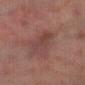Part of a total-body skin-imaging series; this lesion was reviewed on a skin check and was not flagged for biopsy. The recorded lesion diameter is about 4.5 mm. Imaged with cross-polarized lighting. The lesion is on the right forearm. A male patient in their mid-60s. Automated image analysis of the tile measured a footprint of about 8 mm² and two-axis asymmetry of about 0.3. And it measured a mean CIELAB color near L≈37 a*≈19 b*≈20. And it measured a border-irregularity index near 3.5/10. Cropped from a total-body skin-imaging series; the visible field is about 15 mm.